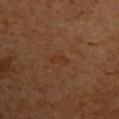Captured during whole-body skin photography for melanoma surveillance; the lesion was not biopsied. Approximately 2.5 mm at its widest. A female patient approximately 50 years of age. From the back. Captured under cross-polarized illumination. A 15 mm close-up extracted from a 3D total-body photography capture.